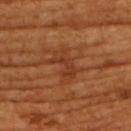notes: total-body-photography surveillance lesion; no biopsy
image source: total-body-photography crop, ~15 mm field of view
diameter: ~3.5 mm (longest diameter)
subject: female, about 55 years old
tile lighting: cross-polarized illumination
site: the back
image-analysis metrics: an area of roughly 5.5 mm², a shape eccentricity near 0.8, and a symmetry-axis asymmetry near 0.45; a lesion color around L≈39 a*≈28 b*≈36 in CIELAB, about 7 CIELAB-L* units darker than the surrounding skin, and a normalized lesion–skin contrast near 6; an automated nevus-likeness rating near 0 out of 100 and lesion-presence confidence of about 95/100